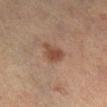biopsy status — imaged on a skin check; not biopsied | body site — the right lower leg | image-analysis metrics — an eccentricity of roughly 0.4 and a shape-asymmetry score of about 0.35 (0 = symmetric); a mean CIELAB color near L≈36 a*≈17 b*≈24, about 8 CIELAB-L* units darker than the surrounding skin, and a lesion-to-skin contrast of about 7.5 (normalized; higher = more distinct) | tile lighting — cross-polarized illumination | image source — 15 mm crop, total-body photography | patient — female, approximately 55 years of age | lesion size — ~3 mm (longest diameter).